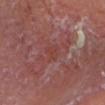From the head or neck. An algorithmic analysis of the crop reported an area of roughly 3 mm², a shape eccentricity near 0.75, and two-axis asymmetry of about 0.45. And it measured a border-irregularity index near 4.5/10, internal color variation of about 1 on a 0–10 scale, and radial color variation of about 0.5. The analysis additionally found a lesion-detection confidence of about 95/100. Imaged with cross-polarized lighting. A male subject aged approximately 65. Cropped from a whole-body photographic skin survey; the tile spans about 15 mm. About 2.5 mm across.